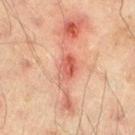{
  "biopsy_status": "not biopsied; imaged during a skin examination",
  "image": {
    "source": "total-body photography crop",
    "field_of_view_mm": 15
  },
  "site": "left lower leg",
  "patient": {
    "sex": "male",
    "age_approx": 45
  },
  "lighting": "cross-polarized"
}